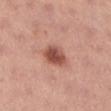Impression:
No biopsy was performed on this lesion — it was imaged during a full skin examination and was not determined to be concerning.
Context:
A region of skin cropped from a whole-body photographic capture, roughly 15 mm wide. The lesion is located on the left lower leg. The recorded lesion diameter is about 4 mm. A female subject, approximately 25 years of age.